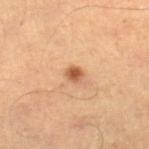notes: imaged on a skin check; not biopsied
automated metrics: a lesion color around L≈54 a*≈24 b*≈36 in CIELAB and a lesion-to-skin contrast of about 9 (normalized; higher = more distinct); border irregularity of about 2 on a 0–10 scale, internal color variation of about 2.5 on a 0–10 scale, and radial color variation of about 0.5
body site: the right lower leg
size: ~2 mm (longest diameter)
patient: male, aged 63–67
lighting: cross-polarized
image source: ~15 mm tile from a whole-body skin photo